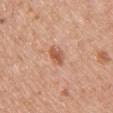Q: Was this lesion biopsied?
A: no biopsy performed (imaged during a skin exam)
Q: Who is the patient?
A: female, approximately 50 years of age
Q: What is the imaging modality?
A: 15 mm crop, total-body photography
Q: Lesion location?
A: the chest